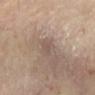{
  "biopsy_status": "not biopsied; imaged during a skin examination"
}